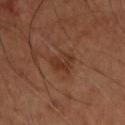{
  "biopsy_status": "not biopsied; imaged during a skin examination",
  "automated_metrics": {
    "area_mm2_approx": 6.5,
    "eccentricity": 0.75,
    "shape_asymmetry": 0.25
  },
  "patient": {
    "sex": "male",
    "age_approx": 55
  },
  "image": {
    "source": "total-body photography crop",
    "field_of_view_mm": 15
  },
  "lighting": "cross-polarized",
  "lesion_size": {
    "long_diameter_mm_approx": 3.5
  },
  "site": "chest"
}